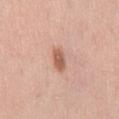The lesion was tiled from a total-body skin photograph and was not biopsied. The total-body-photography lesion software estimated an area of roughly 4 mm², an outline eccentricity of about 0.85 (0 = round, 1 = elongated), and two-axis asymmetry of about 0.2. The software also gave a lesion color around L≈59 a*≈23 b*≈31 in CIELAB, a lesion–skin lightness drop of about 12, and a normalized lesion–skin contrast near 8.5. And it measured a border-irregularity rating of about 2/10, internal color variation of about 2 on a 0–10 scale, and radial color variation of about 1. And it measured a classifier nevus-likeness of about 95/100 and a lesion-detection confidence of about 100/100. A 15 mm close-up extracted from a 3D total-body photography capture. The patient is a male about 40 years old. Located on the mid back. This is a white-light tile.